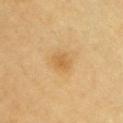A male patient aged 58 to 62.
A 15 mm close-up extracted from a 3D total-body photography capture.
The lesion is located on the chest.